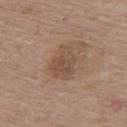Part of a total-body skin-imaging series; this lesion was reviewed on a skin check and was not flagged for biopsy.
A female subject, aged 58–62.
Captured under white-light illumination.
Automated image analysis of the tile measured a mean CIELAB color near L≈49 a*≈16 b*≈28, a lesion–skin lightness drop of about 8, and a normalized lesion–skin contrast near 6.
Longest diameter approximately 4 mm.
A lesion tile, about 15 mm wide, cut from a 3D total-body photograph.
Located on the upper back.